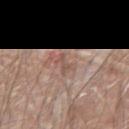notes: total-body-photography surveillance lesion; no biopsy | anatomic site: the left forearm | image: ~15 mm crop, total-body skin-cancer survey | tile lighting: white-light | patient: male, aged 58–62.